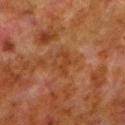image=~15 mm tile from a whole-body skin photo | patient=male, approximately 80 years of age | anatomic site=the left lower leg | diameter=about 3.5 mm | automated metrics=radial color variation of about 1; a nevus-likeness score of about 0/100 and lesion-presence confidence of about 100/100 | tile lighting=cross-polarized illumination.